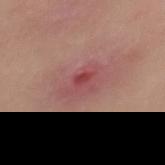This lesion was catalogued during total-body skin photography and was not selected for biopsy. A close-up tile cropped from a whole-body skin photograph, about 15 mm across. A female subject, aged around 25. On the mid back. Longest diameter approximately 4 mm. Captured under white-light illumination.